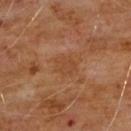follow-up: imaged on a skin check; not biopsied | body site: the chest | patient: male, aged 58–62 | imaging modality: ~15 mm tile from a whole-body skin photo | illumination: cross-polarized | diameter: ≈3 mm.